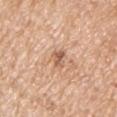{"biopsy_status": "not biopsied; imaged during a skin examination", "image": {"source": "total-body photography crop", "field_of_view_mm": 15}, "lesion_size": {"long_diameter_mm_approx": 2.5}, "patient": {"sex": "male", "age_approx": 65}, "site": "arm", "automated_metrics": {"area_mm2_approx": 3.0, "eccentricity": 0.85, "shape_asymmetry": 0.4, "border_irregularity_0_10": 3.5, "color_variation_0_10": 3.0, "peripheral_color_asymmetry": 1.0}, "lighting": "white-light"}